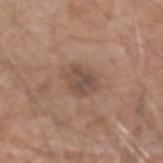No biopsy was performed on this lesion — it was imaged during a full skin examination and was not determined to be concerning. The lesion is on the left forearm. Measured at roughly 3.5 mm in maximum diameter. The tile uses white-light illumination. The patient is a male aged 78 to 82. A region of skin cropped from a whole-body photographic capture, roughly 15 mm wide. The total-body-photography lesion software estimated an outline eccentricity of about 0.55 (0 = round, 1 = elongated) and two-axis asymmetry of about 0.2. It also reported a lesion color around L≈48 a*≈17 b*≈25 in CIELAB, about 9 CIELAB-L* units darker than the surrounding skin, and a normalized border contrast of about 7. And it measured a border-irregularity rating of about 2/10, a within-lesion color-variation index near 3/10, and peripheral color asymmetry of about 1. The analysis additionally found an automated nevus-likeness rating near 15 out of 100 and a detector confidence of about 100 out of 100 that the crop contains a lesion.